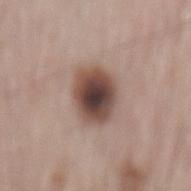follow-up: catalogued during a skin exam; not biopsied | lesion size: ≈5 mm | image: 15 mm crop, total-body photography | automated lesion analysis: an area of roughly 14 mm²; an average lesion color of about L≈46 a*≈17 b*≈22 (CIELAB), a lesion–skin lightness drop of about 17, and a lesion-to-skin contrast of about 12 (normalized; higher = more distinct); a border-irregularity index near 1.5/10 and peripheral color asymmetry of about 1.5 | site: the mid back | subject: male, aged 63 to 67.